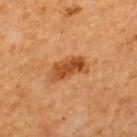Impression:
Captured during whole-body skin photography for melanoma surveillance; the lesion was not biopsied.
Context:
On the upper back. A male patient, aged around 65. A lesion tile, about 15 mm wide, cut from a 3D total-body photograph. The total-body-photography lesion software estimated a lesion area of about 8.5 mm². And it measured an average lesion color of about L≈43 a*≈24 b*≈37 (CIELAB), about 11 CIELAB-L* units darker than the surrounding skin, and a lesion-to-skin contrast of about 8.5 (normalized; higher = more distinct). The software also gave a border-irregularity rating of about 3.5/10, a color-variation rating of about 4/10, and radial color variation of about 1.5. The tile uses cross-polarized illumination. The recorded lesion diameter is about 4.5 mm.